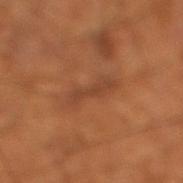Part of a total-body skin-imaging series; this lesion was reviewed on a skin check and was not flagged for biopsy. An algorithmic analysis of the crop reported a border-irregularity index near 5.5/10. The tile uses cross-polarized illumination. A 15 mm close-up extracted from a 3D total-body photography capture. The lesion is on the left leg. The patient is a male in their mid-60s. About 5.5 mm across.